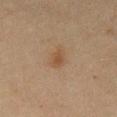Case summary:
– follow-up — catalogued during a skin exam; not biopsied
– TBP lesion metrics — a footprint of about 4 mm², an outline eccentricity of about 0.8 (0 = round, 1 = elongated), and a symmetry-axis asymmetry near 0.2; a mean CIELAB color near L≈40 a*≈15 b*≈28; a classifier nevus-likeness of about 65/100 and lesion-presence confidence of about 100/100
– acquisition — ~15 mm crop, total-body skin-cancer survey
– location — the chest
– illumination — cross-polarized illumination
– diameter — ~3 mm (longest diameter)
– subject — male, about 70 years old